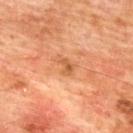Impression: No biopsy was performed on this lesion — it was imaged during a full skin examination and was not determined to be concerning. Background: The total-body-photography lesion software estimated a lesion area of about 3 mm² and two-axis asymmetry of about 0.45. A lesion tile, about 15 mm wide, cut from a 3D total-body photograph. Located on the upper back. About 2.5 mm across. A male subject, in their mid-70s.